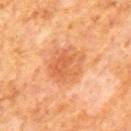{
  "biopsy_status": "not biopsied; imaged during a skin examination",
  "site": "mid back",
  "image": {
    "source": "total-body photography crop",
    "field_of_view_mm": 15
  },
  "patient": {
    "sex": "male",
    "age_approx": 65
  }
}